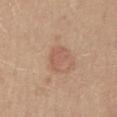The lesion was photographed on a routine skin check and not biopsied; there is no pathology result. The lesion is on the abdomen. Longest diameter approximately 3 mm. A female subject aged around 45. A region of skin cropped from a whole-body photographic capture, roughly 15 mm wide.